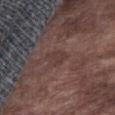Notes:
– workup — total-body-photography surveillance lesion; no biopsy
– illumination — white-light illumination
– location — the abdomen
– acquisition — ~15 mm tile from a whole-body skin photo
– patient — male, roughly 75 years of age